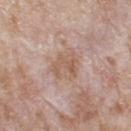Assessment: No biopsy was performed on this lesion — it was imaged during a full skin examination and was not determined to be concerning. Context: Located on the upper back. A roughly 15 mm field-of-view crop from a total-body skin photograph. The subject is a male aged around 80.